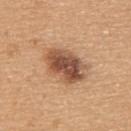Findings:
- follow-up: no biopsy performed (imaged during a skin exam)
- body site: the upper back
- automated lesion analysis: a peripheral color-asymmetry measure near 2
- diameter: ~5.5 mm (longest diameter)
- acquisition: ~15 mm crop, total-body skin-cancer survey
- subject: male, aged around 40
- tile lighting: white-light